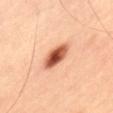| key | value |
|---|---|
| follow-up | imaged on a skin check; not biopsied |
| diameter | ≈4 mm |
| illumination | cross-polarized illumination |
| patient | male, aged 53–57 |
| location | the leg |
| acquisition | 15 mm crop, total-body photography |